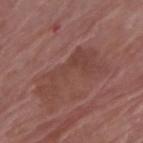Q: How was this image acquired?
A: 15 mm crop, total-body photography
Q: Illumination type?
A: white-light
Q: What is the anatomic site?
A: the right upper arm
Q: Automated lesion metrics?
A: a lesion area of about 15 mm², an eccentricity of roughly 0.9, and a symmetry-axis asymmetry near 0.65; a mean CIELAB color near L≈42 a*≈22 b*≈23, a lesion–skin lightness drop of about 6, and a normalized border contrast of about 5
Q: What are the patient's age and sex?
A: female, approximately 70 years of age
Q: How large is the lesion?
A: ~7 mm (longest diameter)